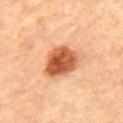Notes:
• workup: imaged on a skin check; not biopsied
• patient: female, aged approximately 65
• image: ~15 mm crop, total-body skin-cancer survey
• body site: the left thigh
• automated metrics: an area of roughly 13 mm² and a symmetry-axis asymmetry near 0.2; a mean CIELAB color near L≈56 a*≈29 b*≈39, roughly 18 lightness units darker than nearby skin, and a normalized border contrast of about 11
• illumination: cross-polarized